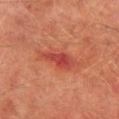follow-up = imaged on a skin check; not biopsied | location = the left thigh | subject = male, in their mid-70s | acquisition = total-body-photography crop, ~15 mm field of view.